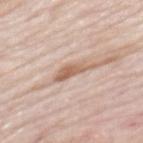A close-up tile cropped from a whole-body skin photograph, about 15 mm across. The subject is a male aged around 85 or older. From the mid back.